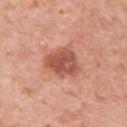| key | value |
|---|---|
| body site | the left upper arm |
| lesion diameter | about 4.5 mm |
| subject | female, aged around 40 |
| lighting | white-light |
| image source | ~15 mm crop, total-body skin-cancer survey |
| automated lesion analysis | a footprint of about 12 mm², a shape eccentricity near 0.6, and a symmetry-axis asymmetry near 0.25; a border-irregularity index near 2.5/10, a within-lesion color-variation index near 5/10, and peripheral color asymmetry of about 1.5; a lesion-detection confidence of about 100/100 |